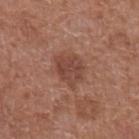{"biopsy_status": "not biopsied; imaged during a skin examination"}